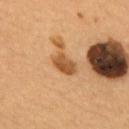The lesion was tiled from a total-body skin photograph and was not biopsied.
The subject is a male aged around 55.
This is a cross-polarized tile.
On the back.
A region of skin cropped from a whole-body photographic capture, roughly 15 mm wide.